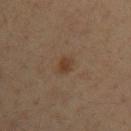Clinical impression: This lesion was catalogued during total-body skin photography and was not selected for biopsy. Acquisition and patient details: Imaged with cross-polarized lighting. The lesion-visualizer software estimated a mean CIELAB color near L≈35 a*≈15 b*≈26 and a lesion-to-skin contrast of about 7 (normalized; higher = more distinct). Cropped from a whole-body photographic skin survey; the tile spans about 15 mm. The recorded lesion diameter is about 2.5 mm. On the left upper arm. A male subject, in their mid-30s.